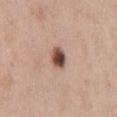| field | value |
|---|---|
| follow-up | catalogued during a skin exam; not biopsied |
| lighting | white-light |
| lesion size | ~2.5 mm (longest diameter) |
| patient | female, approximately 40 years of age |
| imaging modality | 15 mm crop, total-body photography |
| anatomic site | the back |
| automated lesion analysis | a footprint of about 4.5 mm², an outline eccentricity of about 0.7 (0 = round, 1 = elongated), and two-axis asymmetry of about 0.2; a detector confidence of about 100 out of 100 that the crop contains a lesion |